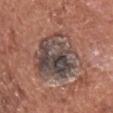notes = no biopsy performed (imaged during a skin exam) | size = ~7.5 mm (longest diameter) | site = the chest | image source = ~15 mm crop, total-body skin-cancer survey | illumination = white-light | subject = male, aged 73 to 77 | automated lesion analysis = a border-irregularity index near 3/10 and radial color variation of about 4.